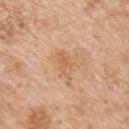| key | value |
|---|---|
| workup | catalogued during a skin exam; not biopsied |
| body site | the right upper arm |
| acquisition | 15 mm crop, total-body photography |
| diameter | about 4.5 mm |
| tile lighting | white-light illumination |
| patient | male, in their mid-60s |
| automated lesion analysis | a symmetry-axis asymmetry near 0.4; border irregularity of about 5 on a 0–10 scale, a color-variation rating of about 3/10, and a peripheral color-asymmetry measure near 1; lesion-presence confidence of about 100/100 |